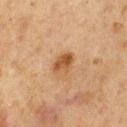No biopsy was performed on this lesion — it was imaged during a full skin examination and was not determined to be concerning. Captured under cross-polarized illumination. A male subject about 75 years old. Measured at roughly 3 mm in maximum diameter. A 15 mm close-up tile from a total-body photography series done for melanoma screening. The lesion is located on the chest.